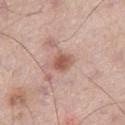Impression: The lesion was photographed on a routine skin check and not biopsied; there is no pathology result. Image and clinical context: The subject is a male in their mid-60s. Imaged with white-light lighting. This image is a 15 mm lesion crop taken from a total-body photograph. From the right thigh.